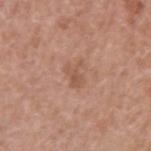biopsy_status: not biopsied; imaged during a skin examination
lighting: white-light
automated_metrics:
  area_mm2_approx: 3.5
  eccentricity: 0.65
  shape_asymmetry: 0.45
  cielab_L: 54
  cielab_a: 22
  cielab_b: 30
  vs_skin_darker_L: 7.0
  vs_skin_contrast_norm: 5.5
  color_variation_0_10: 1.5
  peripheral_color_asymmetry: 0.5
  nevus_likeness_0_100: 0
  lesion_detection_confidence_0_100: 100
lesion_size:
  long_diameter_mm_approx: 2.5
site: left upper arm
patient:
  sex: male
  age_approx: 60
image:
  source: total-body photography crop
  field_of_view_mm: 15